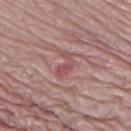<lesion>
<biopsy_status>not biopsied; imaged during a skin examination</biopsy_status>
<lighting>white-light</lighting>
<automated_metrics>
  <eccentricity>0.85</eccentricity>
  <shape_asymmetry>0.6</shape_asymmetry>
  <cielab_L>51</cielab_L>
  <cielab_a>25</cielab_a>
  <cielab_b>20</cielab_b>
  <vs_skin_contrast_norm>6.0</vs_skin_contrast_norm>
  <lesion_detection_confidence_0_100>70</lesion_detection_confidence_0_100>
</automated_metrics>
<site>left thigh</site>
<patient>
  <sex>female</sex>
  <age_approx>70</age_approx>
</patient>
<image>
  <source>total-body photography crop</source>
  <field_of_view_mm>15</field_of_view_mm>
</image>
</lesion>